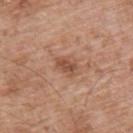Clinical impression: The lesion was photographed on a routine skin check and not biopsied; there is no pathology result. Background: A male subject aged 53 to 57. The lesion is on the upper back. Approximately 3 mm at its widest. Imaged with white-light lighting. This image is a 15 mm lesion crop taken from a total-body photograph.